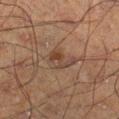No biopsy was performed on this lesion — it was imaged during a full skin examination and was not determined to be concerning.
A male subject, about 50 years old.
This is a cross-polarized tile.
A 15 mm crop from a total-body photograph taken for skin-cancer surveillance.
Approximately 4 mm at its widest.
From the leg.
An algorithmic analysis of the crop reported a mean CIELAB color near L≈33 a*≈14 b*≈22 and a normalized lesion–skin contrast near 6. The analysis additionally found a border-irregularity index near 4/10 and a color-variation rating of about 5/10. And it measured a lesion-detection confidence of about 95/100.